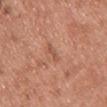Findings:
- site — the chest
- tile lighting — white-light
- acquisition — ~15 mm crop, total-body skin-cancer survey
- patient — female, aged 48–52